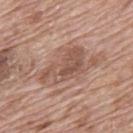Captured during whole-body skin photography for melanoma surveillance; the lesion was not biopsied.
The lesion-visualizer software estimated an area of roughly 13 mm², an eccentricity of roughly 0.85, and a symmetry-axis asymmetry near 0.4.
The lesion is on the back.
A close-up tile cropped from a whole-body skin photograph, about 15 mm across.
The lesion's longest dimension is about 6 mm.
A male patient, aged 68–72.
The tile uses white-light illumination.